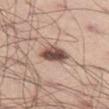Captured during whole-body skin photography for melanoma surveillance; the lesion was not biopsied.
The lesion is located on the left thigh.
The lesion-visualizer software estimated a footprint of about 7.5 mm² and a shape-asymmetry score of about 0.25 (0 = symmetric). And it measured a classifier nevus-likeness of about 90/100 and a lesion-detection confidence of about 100/100.
The lesion's longest dimension is about 4 mm.
A 15 mm close-up extracted from a 3D total-body photography capture.
A male subject approximately 45 years of age.